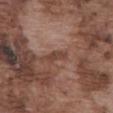Notes:
* follow-up: total-body-photography surveillance lesion; no biopsy
* patient: male, roughly 75 years of age
* lighting: white-light illumination
* anatomic site: the abdomen
* image: ~15 mm crop, total-body skin-cancer survey
* size: ~3 mm (longest diameter)
* automated metrics: a footprint of about 3 mm², an eccentricity of roughly 0.9, and a shape-asymmetry score of about 0.3 (0 = symmetric); an average lesion color of about L≈42 a*≈20 b*≈25 (CIELAB), about 8 CIELAB-L* units darker than the surrounding skin, and a lesion-to-skin contrast of about 7 (normalized; higher = more distinct); a border-irregularity rating of about 3/10, a within-lesion color-variation index near 1/10, and peripheral color asymmetry of about 0.5